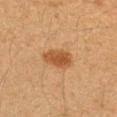{
  "biopsy_status": "not biopsied; imaged during a skin examination",
  "lesion_size": {
    "long_diameter_mm_approx": 4.0
  },
  "image": {
    "source": "total-body photography crop",
    "field_of_view_mm": 15
  },
  "lighting": "cross-polarized",
  "patient": {
    "sex": "female",
    "age_approx": 40
  },
  "site": "left upper arm"
}